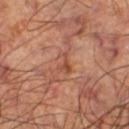follow-up — no biopsy performed (imaged during a skin exam)
body site — the right thigh
size — ≈3 mm
automated metrics — a footprint of about 3 mm² and a shape eccentricity near 0.85; a lesion color around L≈47 a*≈25 b*≈31 in CIELAB and a lesion–skin lightness drop of about 8
illumination — cross-polarized
imaging modality — total-body-photography crop, ~15 mm field of view
subject — male, aged around 60